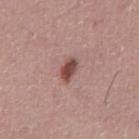automated metrics: a mean CIELAB color near L≈48 a*≈21 b*≈23, about 13 CIELAB-L* units darker than the surrounding skin, and a normalized border contrast of about 9.5 | site: the front of the torso | subject: male, aged 43 to 47 | tile lighting: white-light illumination | lesion diameter: about 3 mm | image: ~15 mm tile from a whole-body skin photo.